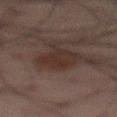workup = no biopsy performed (imaged during a skin exam)
tile lighting = cross-polarized illumination
anatomic site = the lower back
lesion diameter = ≈4.5 mm
image = total-body-photography crop, ~15 mm field of view
patient = male, in their mid- to late 60s